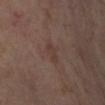subject = female, approximately 55 years of age
tile lighting = cross-polarized illumination
anatomic site = the right lower leg
acquisition = ~15 mm crop, total-body skin-cancer survey
lesion size = ~3 mm (longest diameter)